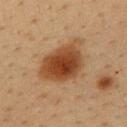biopsy status = total-body-photography surveillance lesion; no biopsy | site = the upper back | automated metrics = an average lesion color of about L≈39 a*≈20 b*≈32 (CIELAB) and roughly 12 lightness units darker than nearby skin | tile lighting = cross-polarized illumination | patient = female, in their 40s | imaging modality = total-body-photography crop, ~15 mm field of view.